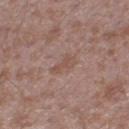biopsy status: imaged on a skin check; not biopsied | illumination: white-light | image source: total-body-photography crop, ~15 mm field of view | lesion diameter: ~3 mm (longest diameter) | body site: the right thigh | subject: male, aged 43 to 47.